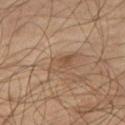<tbp_lesion>
<biopsy_status>not biopsied; imaged during a skin examination</biopsy_status>
<patient>
  <sex>male</sex>
  <age_approx>55</age_approx>
</patient>
<lesion_size>
  <long_diameter_mm_approx>3.5</long_diameter_mm_approx>
</lesion_size>
<site>leg</site>
<lighting>cross-polarized</lighting>
<automated_metrics>
  <vs_skin_darker_L>7.0</vs_skin_darker_L>
  <border_irregularity_0_10>4.0</border_irregularity_0_10>
  <color_variation_0_10>3.5</color_variation_0_10>
</automated_metrics>
<image>
  <source>total-body photography crop</source>
  <field_of_view_mm>15</field_of_view_mm>
</image>
</tbp_lesion>